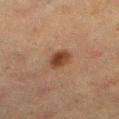A close-up tile cropped from a whole-body skin photograph, about 15 mm across. On the left lower leg. Automated image analysis of the tile measured a lesion area of about 5.5 mm² and two-axis asymmetry of about 0.15. The analysis additionally found a mean CIELAB color near L≈34 a*≈18 b*≈27, roughly 10 lightness units darker than nearby skin, and a normalized border contrast of about 9.5. It also reported a nevus-likeness score of about 100/100 and a lesion-detection confidence of about 100/100. A female patient aged 53 to 57. This is a cross-polarized tile.